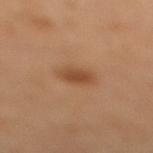{"biopsy_status": "not biopsied; imaged during a skin examination", "image": {"source": "total-body photography crop", "field_of_view_mm": 15}, "patient": {"sex": "female", "age_approx": 60}, "site": "right lower leg"}